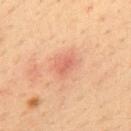The lesion was photographed on a routine skin check and not biopsied; there is no pathology result. Longest diameter approximately 2.5 mm. Imaged with cross-polarized lighting. The patient is a male roughly 35 years of age. A lesion tile, about 15 mm wide, cut from a 3D total-body photograph. Located on the upper back.